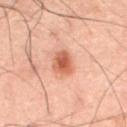Impression:
No biopsy was performed on this lesion — it was imaged during a full skin examination and was not determined to be concerning.
Context:
Approximately 3 mm at its widest. Cropped from a whole-body photographic skin survey; the tile spans about 15 mm. The lesion is located on the abdomen. Captured under cross-polarized illumination. The lesion-visualizer software estimated a lesion–skin lightness drop of about 11. A male subject in their 60s.